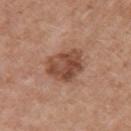The lesion was photographed on a routine skin check and not biopsied; there is no pathology result. A female patient in their 40s. On the left upper arm. A 15 mm close-up extracted from a 3D total-body photography capture.